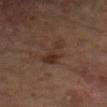  biopsy_status: not biopsied; imaged during a skin examination
  site: left forearm
  patient:
    sex: female
    age_approx: 70
  image:
    source: total-body photography crop
    field_of_view_mm: 15
  lesion_size:
    long_diameter_mm_approx: 4.5
  lighting: cross-polarized